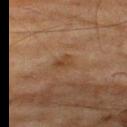Part of a total-body skin-imaging series; this lesion was reviewed on a skin check and was not flagged for biopsy.